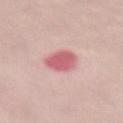<record>
  <biopsy_status>not biopsied; imaged during a skin examination</biopsy_status>
</record>